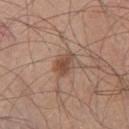Recorded during total-body skin imaging; not selected for excision or biopsy. The lesion is located on the left thigh. A male patient, in their mid-40s. A 15 mm close-up tile from a total-body photography series done for melanoma screening. The total-body-photography lesion software estimated a detector confidence of about 100 out of 100 that the crop contains a lesion. Captured under white-light illumination.